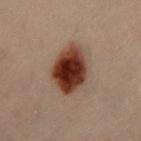Q: Was this lesion biopsied?
A: no biopsy performed (imaged during a skin exam)
Q: Automated lesion metrics?
A: a lesion area of about 18 mm², a shape eccentricity near 0.8, and two-axis asymmetry of about 0.15
Q: What is the lesion's diameter?
A: ~6.5 mm (longest diameter)
Q: Who is the patient?
A: male, in their 50s
Q: What lighting was used for the tile?
A: cross-polarized
Q: Where on the body is the lesion?
A: the chest
Q: What kind of image is this?
A: ~15 mm tile from a whole-body skin photo